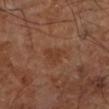follow-up: total-body-photography surveillance lesion; no biopsy | subject: in their mid- to late 60s | image: ~15 mm crop, total-body skin-cancer survey | location: the leg.